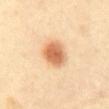Recorded during total-body skin imaging; not selected for excision or biopsy. A close-up tile cropped from a whole-body skin photograph, about 15 mm across. The lesion is located on the mid back. A female subject approximately 40 years of age. The lesion's longest dimension is about 4 mm.